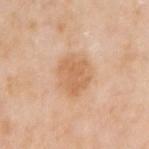workup: imaged on a skin check; not biopsied | imaging modality: ~15 mm tile from a whole-body skin photo | patient: male, in their mid-70s | tile lighting: white-light illumination | lesion size: about 4 mm | site: the right upper arm | automated metrics: an area of roughly 12 mm² and an outline eccentricity of about 0.4 (0 = round, 1 = elongated); a mean CIELAB color near L≈64 a*≈21 b*≈37, a lesion–skin lightness drop of about 9, and a normalized lesion–skin contrast near 6.5.